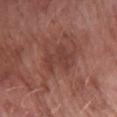{
  "biopsy_status": "not biopsied; imaged during a skin examination",
  "image": {
    "source": "total-body photography crop",
    "field_of_view_mm": 15
  },
  "automated_metrics": {
    "nevus_likeness_0_100": 0,
    "lesion_detection_confidence_0_100": 100
  },
  "lighting": "white-light",
  "lesion_size": {
    "long_diameter_mm_approx": 4.0
  },
  "patient": {
    "sex": "female",
    "age_approx": 60
  },
  "site": "right forearm"
}